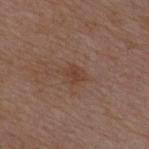Impression:
Imaged during a routine full-body skin examination; the lesion was not biopsied and no histopathology is available.
Image and clinical context:
A male patient, aged around 50. Located on the chest. A 15 mm close-up tile from a total-body photography series done for melanoma screening.